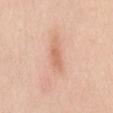Part of a total-body skin-imaging series; this lesion was reviewed on a skin check and was not flagged for biopsy. The lesion is located on the mid back. A region of skin cropped from a whole-body photographic capture, roughly 15 mm wide. Imaged with white-light lighting. About 4.5 mm across. A female patient, aged around 40.